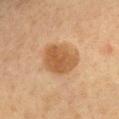Impression: Imaged during a routine full-body skin examination; the lesion was not biopsied and no histopathology is available. Acquisition and patient details: Imaged with cross-polarized lighting. The subject is a female in their 50s. The recorded lesion diameter is about 4.5 mm. This image is a 15 mm lesion crop taken from a total-body photograph. The lesion is located on the chest. The lesion-visualizer software estimated an automated nevus-likeness rating near 75 out of 100.